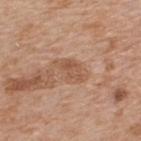Q: Is there a histopathology result?
A: total-body-photography surveillance lesion; no biopsy
Q: How large is the lesion?
A: ~3.5 mm (longest diameter)
Q: Where on the body is the lesion?
A: the back
Q: How was this image acquired?
A: 15 mm crop, total-body photography
Q: Patient demographics?
A: male, aged approximately 60